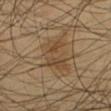Captured during whole-body skin photography for melanoma surveillance; the lesion was not biopsied.
The lesion is located on the left lower leg.
Measured at roughly 5 mm in maximum diameter.
A male patient aged 63–67.
This is a cross-polarized tile.
Cropped from a total-body skin-imaging series; the visible field is about 15 mm.